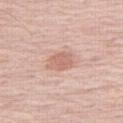Clinical summary: A female patient, about 60 years old. The lesion is located on the leg. This image is a 15 mm lesion crop taken from a total-body photograph. The lesion-visualizer software estimated a footprint of about 6 mm² and two-axis asymmetry of about 0.25. It also reported a lesion color around L≈64 a*≈22 b*≈28 in CIELAB, about 9 CIELAB-L* units darker than the surrounding skin, and a normalized lesion–skin contrast near 6. The analysis additionally found border irregularity of about 2.5 on a 0–10 scale. Measured at roughly 3 mm in maximum diameter. This is a white-light tile.